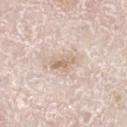Q: Was this lesion biopsied?
A: no biopsy performed (imaged during a skin exam)
Q: Where on the body is the lesion?
A: the left lower leg
Q: Illumination type?
A: white-light illumination
Q: What are the patient's age and sex?
A: male, aged 78–82
Q: What kind of image is this?
A: 15 mm crop, total-body photography
Q: What did automated image analysis measure?
A: a shape eccentricity near 0.8; radial color variation of about 1.5